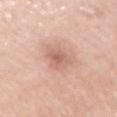Q: Was a biopsy performed?
A: imaged on a skin check; not biopsied
Q: What is the anatomic site?
A: the right upper arm
Q: Lesion size?
A: ≈3.5 mm
Q: What are the patient's age and sex?
A: female, aged around 65
Q: What kind of image is this?
A: ~15 mm tile from a whole-body skin photo